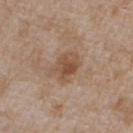notes=total-body-photography surveillance lesion; no biopsy | body site=the chest | lesion size=about 3.5 mm | image=~15 mm crop, total-body skin-cancer survey | subject=male, aged 63 to 67 | tile lighting=white-light illumination.